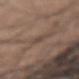<lesion>
  <biopsy_status>not biopsied; imaged during a skin examination</biopsy_status>
  <patient>
    <sex>male</sex>
    <age_approx>35</age_approx>
  </patient>
  <automated_metrics>
    <eccentricity>0.9</eccentricity>
    <shape_asymmetry>0.35</shape_asymmetry>
    <border_irregularity_0_10>3.5</border_irregularity_0_10>
    <color_variation_0_10>0.0</color_variation_0_10>
  </automated_metrics>
  <lesion_size>
    <long_diameter_mm_approx>2.5</long_diameter_mm_approx>
  </lesion_size>
  <image>
    <source>total-body photography crop</source>
    <field_of_view_mm>15</field_of_view_mm>
  </image>
  <site>right upper arm</site>
</lesion>